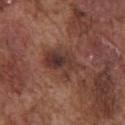Case summary:
• biopsy status · catalogued during a skin exam; not biopsied